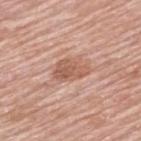Clinical summary:
The lesion-visualizer software estimated about 10 CIELAB-L* units darker than the surrounding skin and a normalized lesion–skin contrast near 7. And it measured a nevus-likeness score of about 80/100 and a detector confidence of about 100 out of 100 that the crop contains a lesion. A roughly 15 mm field-of-view crop from a total-body skin photograph. About 4 mm across. The subject is a male aged approximately 80. Located on the back. This is a white-light tile.